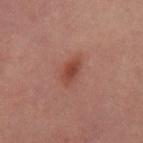Recorded during total-body skin imaging; not selected for excision or biopsy.
On the right thigh.
A female subject about 40 years old.
A 15 mm close-up extracted from a 3D total-body photography capture.